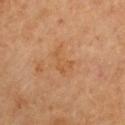Findings:
- biopsy status — total-body-photography surveillance lesion; no biopsy
- location — the left arm
- patient — male, in their mid- to late 60s
- lesion size — about 3.5 mm
- image — 15 mm crop, total-body photography
- tile lighting — cross-polarized